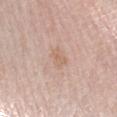subject: female, aged 58 to 62; location: the left forearm; image: 15 mm crop, total-body photography.